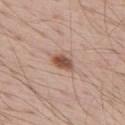Q: What is the imaging modality?
A: ~15 mm crop, total-body skin-cancer survey
Q: How was the tile lit?
A: white-light illumination
Q: Where on the body is the lesion?
A: the back
Q: What are the patient's age and sex?
A: male, aged approximately 70
Q: How large is the lesion?
A: ~3 mm (longest diameter)
Q: Automated lesion metrics?
A: an automated nevus-likeness rating near 95 out of 100